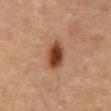Assessment: No biopsy was performed on this lesion — it was imaged during a full skin examination and was not determined to be concerning.